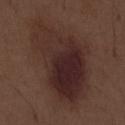Part of a total-body skin-imaging series; this lesion was reviewed on a skin check and was not flagged for biopsy.
The lesion's longest dimension is about 11.5 mm.
On the abdomen.
A 15 mm close-up tile from a total-body photography series done for melanoma screening.
A male subject, aged 68 to 72.
The lesion-visualizer software estimated a lesion area of about 49 mm². The analysis additionally found a within-lesion color-variation index near 7/10 and peripheral color asymmetry of about 2. The analysis additionally found an automated nevus-likeness rating near 60 out of 100 and lesion-presence confidence of about 100/100.